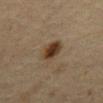Assessment: The lesion was tiled from a total-body skin photograph and was not biopsied. Clinical summary: The lesion is on the mid back. Approximately 3 mm at its widest. A male subject, roughly 60 years of age. A roughly 15 mm field-of-view crop from a total-body skin photograph.